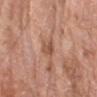This lesion was catalogued during total-body skin photography and was not selected for biopsy. The lesion is located on the right forearm. A roughly 15 mm field-of-view crop from a total-body skin photograph. A male patient approximately 80 years of age.